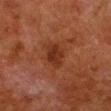{"biopsy_status": "not biopsied; imaged during a skin examination", "lesion_size": {"long_diameter_mm_approx": 3.5}, "automated_metrics": {"cielab_L": 25, "cielab_a": 22, "cielab_b": 26, "vs_skin_darker_L": 7.0, "vs_skin_contrast_norm": 7.5, "border_irregularity_0_10": 2.0, "color_variation_0_10": 2.0, "peripheral_color_asymmetry": 1.0}, "lighting": "cross-polarized", "patient": {"sex": "male", "age_approx": 80}, "image": {"source": "total-body photography crop", "field_of_view_mm": 15}, "site": "left lower leg"}